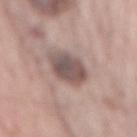biopsy status: imaged on a skin check; not biopsied
body site: the back
patient: male, in their mid-60s
image-analysis metrics: a footprint of about 11 mm², an outline eccentricity of about 0.75 (0 = round, 1 = elongated), and two-axis asymmetry of about 0.15; an average lesion color of about L≈51 a*≈15 b*≈19 (CIELAB), roughly 13 lightness units darker than nearby skin, and a normalized border contrast of about 9.5; a nevus-likeness score of about 10/100
image source: 15 mm crop, total-body photography
illumination: white-light